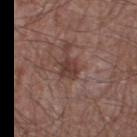biopsy_status: not biopsied; imaged during a skin examination
lighting: white-light
site: right thigh
patient:
  sex: male
  age_approx: 60
image:
  source: total-body photography crop
  field_of_view_mm: 15